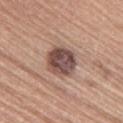Findings:
– biopsy status · imaged on a skin check; not biopsied
– automated metrics · a lesion color around L≈48 a*≈19 b*≈22 in CIELAB, about 15 CIELAB-L* units darker than the surrounding skin, and a normalized lesion–skin contrast near 11
– subject · female, in their mid- to late 60s
– illumination · white-light illumination
– site · the upper back
– imaging modality · ~15 mm crop, total-body skin-cancer survey